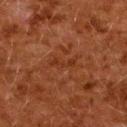Impression:
Part of a total-body skin-imaging series; this lesion was reviewed on a skin check and was not flagged for biopsy.
Clinical summary:
Imaged with cross-polarized lighting. A roughly 15 mm field-of-view crop from a total-body skin photograph. From the upper back. An algorithmic analysis of the crop reported an average lesion color of about L≈27 a*≈23 b*≈30 (CIELAB), a lesion–skin lightness drop of about 5, and a lesion-to-skin contrast of about 5.5 (normalized; higher = more distinct). It also reported a nevus-likeness score of about 0/100 and lesion-presence confidence of about 100/100. A female patient, about 50 years old.